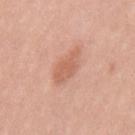Image and clinical context: A lesion tile, about 15 mm wide, cut from a 3D total-body photograph. Located on the mid back. A male patient in their mid- to late 40s. Approximately 4.5 mm at its widest. Automated tile analysis of the lesion measured an area of roughly 7.5 mm², a shape eccentricity near 0.85, and a shape-asymmetry score of about 0.25 (0 = symmetric). The software also gave about 9 CIELAB-L* units darker than the surrounding skin and a lesion-to-skin contrast of about 6 (normalized; higher = more distinct). Imaged with white-light lighting.